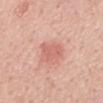{
  "biopsy_status": "not biopsied; imaged during a skin examination",
  "lighting": "white-light",
  "automated_metrics": {
    "border_irregularity_0_10": 3.5,
    "peripheral_color_asymmetry": 0.5,
    "lesion_detection_confidence_0_100": 100
  },
  "image": {
    "source": "total-body photography crop",
    "field_of_view_mm": 15
  },
  "lesion_size": {
    "long_diameter_mm_approx": 3.5
  },
  "patient": {
    "sex": "male",
    "age_approx": 55
  },
  "site": "mid back"
}